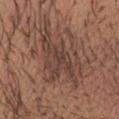biopsy_status: not biopsied; imaged during a skin examination
lesion_size:
  long_diameter_mm_approx: 9.0
patient:
  sex: female
  age_approx: 25
site: abdomen
image:
  source: total-body photography crop
  field_of_view_mm: 15
automated_metrics:
  area_mm2_approx: 23.0
  eccentricity: 0.85
  shape_asymmetry: 0.5